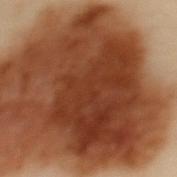Case summary:
- notes · imaged on a skin check; not biopsied
- illumination · cross-polarized illumination
- lesion size · ~15 mm (longest diameter)
- acquisition · total-body-photography crop, ~15 mm field of view
- body site · the upper back
- patient · female, roughly 60 years of age